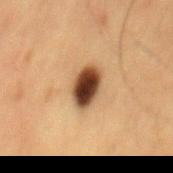A male subject aged 58 to 62.
A roughly 15 mm field-of-view crop from a total-body skin photograph.
Longest diameter approximately 4 mm.
Captured under cross-polarized illumination.
The lesion-visualizer software estimated a footprint of about 8.5 mm² and an eccentricity of roughly 0.75. The software also gave a border-irregularity index near 1.5/10, a within-lesion color-variation index near 8/10, and a peripheral color-asymmetry measure near 2.5. The software also gave an automated nevus-likeness rating near 100 out of 100 and a detector confidence of about 100 out of 100 that the crop contains a lesion.